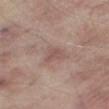This lesion was catalogued during total-body skin photography and was not selected for biopsy.
Longest diameter approximately 3 mm.
From the right thigh.
Imaged with white-light lighting.
A male subject, aged around 65.
A 15 mm close-up extracted from a 3D total-body photography capture.
An algorithmic analysis of the crop reported a border-irregularity rating of about 3.5/10, a color-variation rating of about 2/10, and a peripheral color-asymmetry measure near 0.5. And it measured an automated nevus-likeness rating near 0 out of 100 and a lesion-detection confidence of about 100/100.